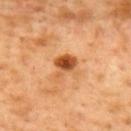notes — no biopsy performed (imaged during a skin exam)
automated lesion analysis — an area of roughly 6 mm² and a symmetry-axis asymmetry near 0.35; a mean CIELAB color near L≈54 a*≈28 b*≈43, roughly 15 lightness units darker than nearby skin, and a normalized lesion–skin contrast near 9.5
lighting — cross-polarized illumination
location — the upper back
acquisition — ~15 mm crop, total-body skin-cancer survey
subject — male, aged approximately 50